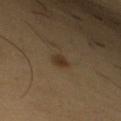follow-up=catalogued during a skin exam; not biopsied | lesion size=~2 mm (longest diameter) | patient=female, in their mid-50s | tile lighting=cross-polarized illumination | anatomic site=the arm | image source=15 mm crop, total-body photography.